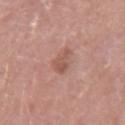Assessment: Part of a total-body skin-imaging series; this lesion was reviewed on a skin check and was not flagged for biopsy. Image and clinical context: A close-up tile cropped from a whole-body skin photograph, about 15 mm across. The lesion is on the right forearm. Longest diameter approximately 3 mm. The tile uses white-light illumination. The patient is a female aged approximately 45.